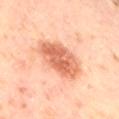Notes:
– workup: catalogued during a skin exam; not biopsied
– image: 15 mm crop, total-body photography
– lighting: cross-polarized illumination
– patient: male, in their mid-60s
– lesion diameter: about 5.5 mm
– body site: the right thigh